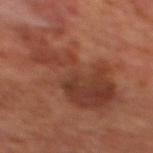site: back
automated_metrics:
  area_mm2_approx: 34.0
  shape_asymmetry: 0.55
  color_variation_0_10: 4.5
  peripheral_color_asymmetry: 1.5
  nevus_likeness_0_100: 0
image:
  source: total-body photography crop
  field_of_view_mm: 15
patient:
  sex: male
  age_approx: 70